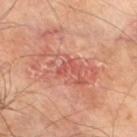Imaged during a routine full-body skin examination; the lesion was not biopsied and no histopathology is available.
A male patient, aged 68–72.
The lesion is located on the right thigh.
Cropped from a whole-body photographic skin survey; the tile spans about 15 mm.